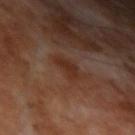Imaged during a routine full-body skin examination; the lesion was not biopsied and no histopathology is available. The lesion's longest dimension is about 3 mm. The total-body-photography lesion software estimated a lesion area of about 4.5 mm², an outline eccentricity of about 0.85 (0 = round, 1 = elongated), and a symmetry-axis asymmetry near 0.35. The software also gave a border-irregularity rating of about 3.5/10, internal color variation of about 1.5 on a 0–10 scale, and a peripheral color-asymmetry measure near 0.5. The software also gave a nevus-likeness score of about 0/100 and a detector confidence of about 100 out of 100 that the crop contains a lesion. A male patient in their 70s. Cropped from a total-body skin-imaging series; the visible field is about 15 mm. On the left upper arm.